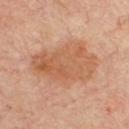<record>
  <biopsy_status>not biopsied; imaged during a skin examination</biopsy_status>
  <patient>
    <sex>male</sex>
    <age_approx>65</age_approx>
  </patient>
  <image>
    <source>total-body photography crop</source>
    <field_of_view_mm>15</field_of_view_mm>
  </image>
  <site>chest</site>
</record>